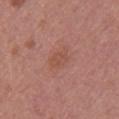notes: no biopsy performed (imaged during a skin exam)
automated lesion analysis: a lesion-detection confidence of about 100/100
lesion size: ~3 mm (longest diameter)
patient: female, aged 48 to 52
body site: the left upper arm
imaging modality: 15 mm crop, total-body photography
lighting: white-light illumination